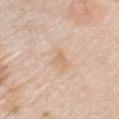The lesion was photographed on a routine skin check and not biopsied; there is no pathology result.
Cropped from a whole-body photographic skin survey; the tile spans about 15 mm.
A male patient, in their mid-70s.
Measured at roughly 2.5 mm in maximum diameter.
This is a white-light tile.
The lesion-visualizer software estimated a symmetry-axis asymmetry near 0.55. And it measured about 6 CIELAB-L* units darker than the surrounding skin and a normalized lesion–skin contrast near 5. The analysis additionally found a border-irregularity rating of about 5/10 and a within-lesion color-variation index near 1/10. The analysis additionally found a detector confidence of about 100 out of 100 that the crop contains a lesion.
Located on the left upper arm.